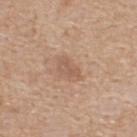{
  "biopsy_status": "not biopsied; imaged during a skin examination",
  "site": "upper back",
  "patient": {
    "sex": "male",
    "age_approx": 60
  },
  "lighting": "white-light",
  "image": {
    "source": "total-body photography crop",
    "field_of_view_mm": 15
  }
}